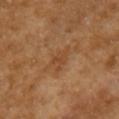{
  "biopsy_status": "not biopsied; imaged during a skin examination",
  "patient": {
    "sex": "female",
    "age_approx": 50
  },
  "lesion_size": {
    "long_diameter_mm_approx": 2.5
  },
  "lighting": "cross-polarized",
  "image": {
    "source": "total-body photography crop",
    "field_of_view_mm": 15
  },
  "site": "left upper arm",
  "automated_metrics": {
    "area_mm2_approx": 3.0,
    "border_irregularity_0_10": 5.5,
    "peripheral_color_asymmetry": 0.5
  }
}